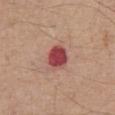image — 15 mm crop, total-body photography | size — ≈3 mm | automated metrics — a lesion color around L≈46 a*≈33 b*≈23 in CIELAB, a lesion–skin lightness drop of about 16, and a lesion-to-skin contrast of about 12 (normalized; higher = more distinct); a border-irregularity index near 1.5/10, internal color variation of about 2.5 on a 0–10 scale, and peripheral color asymmetry of about 1 | subject — male, roughly 75 years of age | location — the abdomen.